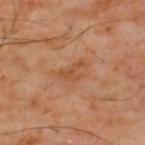workup = catalogued during a skin exam; not biopsied
diameter = ~3.5 mm (longest diameter)
lighting = cross-polarized
image = ~15 mm crop, total-body skin-cancer survey
patient = male, approximately 60 years of age
site = the mid back